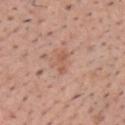| feature | finding |
|---|---|
| follow-up | imaged on a skin check; not biopsied |